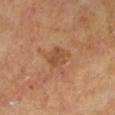<tbp_lesion>
<biopsy_status>not biopsied; imaged during a skin examination</biopsy_status>
<patient>
  <sex>male</sex>
  <age_approx>65</age_approx>
</patient>
<lesion_size>
  <long_diameter_mm_approx>3.0</long_diameter_mm_approx>
</lesion_size>
<image>
  <source>total-body photography crop</source>
  <field_of_view_mm>15</field_of_view_mm>
</image>
<automated_metrics>
  <cielab_L>47</cielab_L>
  <cielab_a>21</cielab_a>
  <cielab_b>33</cielab_b>
  <vs_skin_darker_L>7.0</vs_skin_darker_L>
  <nevus_likeness_0_100>0</nevus_likeness_0_100>
  <lesion_detection_confidence_0_100>100</lesion_detection_confidence_0_100>
</automated_metrics>
<lighting>cross-polarized</lighting>
<site>left lower leg</site>
</tbp_lesion>